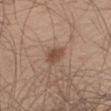Clinical impression:
Recorded during total-body skin imaging; not selected for excision or biopsy.
Image and clinical context:
This is a white-light tile. A close-up tile cropped from a whole-body skin photograph, about 15 mm across. An algorithmic analysis of the crop reported a lesion area of about 4.5 mm² and a symmetry-axis asymmetry near 0.25. The analysis additionally found about 10 CIELAB-L* units darker than the surrounding skin and a normalized lesion–skin contrast near 7.5. And it measured a border-irregularity rating of about 2.5/10. The lesion's longest dimension is about 3 mm. A male subject in their 60s. The lesion is located on the right thigh.